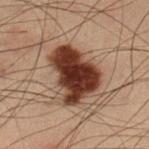biopsy status: total-body-photography surveillance lesion; no biopsy | automated metrics: a footprint of about 22 mm², an outline eccentricity of about 0.7 (0 = round, 1 = elongated), and a shape-asymmetry score of about 0.3 (0 = symmetric); a lesion color around L≈29 a*≈19 b*≈23 in CIELAB and roughly 18 lightness units darker than nearby skin; a color-variation rating of about 5/10; lesion-presence confidence of about 100/100 | patient: male, about 55 years old | location: the left lower leg | image source: ~15 mm tile from a whole-body skin photo | diameter: about 6.5 mm | illumination: cross-polarized.